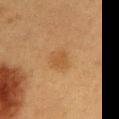Assessment: Recorded during total-body skin imaging; not selected for excision or biopsy. Background: The lesion is on the chest. A 15 mm close-up extracted from a 3D total-body photography capture. Captured under cross-polarized illumination. The patient is a female roughly 40 years of age. The recorded lesion diameter is about 3 mm.